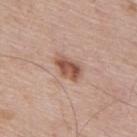workup: no biopsy performed (imaged during a skin exam) | patient: male, aged around 65 | automated lesion analysis: an area of roughly 7 mm², a shape eccentricity near 0.8, and a shape-asymmetry score of about 0.2 (0 = symmetric); roughly 13 lightness units darker than nearby skin and a normalized lesion–skin contrast near 9; a classifier nevus-likeness of about 90/100 and lesion-presence confidence of about 100/100 | anatomic site: the back | image source: ~15 mm crop, total-body skin-cancer survey | lesion size: ~3.5 mm (longest diameter) | tile lighting: white-light.